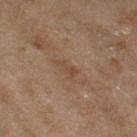Notes:
* imaging modality — total-body-photography crop, ~15 mm field of view
* subject — female, aged 58 to 62
* location — the right thigh